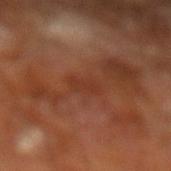acquisition=total-body-photography crop, ~15 mm field of view
anatomic site=the left lower leg
subject=male, in their mid-60s